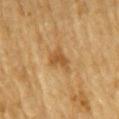Q: Is there a histopathology result?
A: total-body-photography surveillance lesion; no biopsy
Q: What is the imaging modality?
A: ~15 mm tile from a whole-body skin photo
Q: How large is the lesion?
A: about 3 mm
Q: How was the tile lit?
A: cross-polarized
Q: What did automated image analysis measure?
A: a lesion area of about 5 mm² and an eccentricity of roughly 0.7; a color-variation rating of about 2.5/10 and a peripheral color-asymmetry measure near 1; a classifier nevus-likeness of about 20/100 and lesion-presence confidence of about 100/100
Q: What are the patient's age and sex?
A: male, about 85 years old or older
Q: Lesion location?
A: the right upper arm